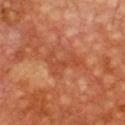Q: Was this lesion biopsied?
A: total-body-photography surveillance lesion; no biopsy
Q: Where on the body is the lesion?
A: the chest
Q: Who is the patient?
A: male, aged 58–62
Q: What kind of image is this?
A: ~15 mm crop, total-body skin-cancer survey
Q: How large is the lesion?
A: ≈4.5 mm